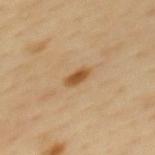{"biopsy_status": "not biopsied; imaged during a skin examination", "lighting": "cross-polarized", "image": {"source": "total-body photography crop", "field_of_view_mm": 15}, "site": "upper back", "lesion_size": {"long_diameter_mm_approx": 2.5}, "automated_metrics": {"area_mm2_approx": 3.5, "eccentricity": 0.8, "shape_asymmetry": 0.25, "cielab_L": 52, "cielab_a": 19, "cielab_b": 39, "vs_skin_darker_L": 11.0, "vs_skin_contrast_norm": 9.0}, "patient": {"sex": "female", "age_approx": 50}}